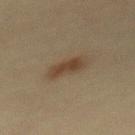Captured during whole-body skin photography for melanoma surveillance; the lesion was not biopsied. Longest diameter approximately 3.5 mm. This is a cross-polarized tile. A region of skin cropped from a whole-body photographic capture, roughly 15 mm wide. On the back. The subject is a female aged approximately 40. The total-body-photography lesion software estimated a mean CIELAB color near L≈34 a*≈13 b*≈25, a lesion–skin lightness drop of about 8, and a normalized border contrast of about 8. The software also gave a color-variation rating of about 1.5/10 and radial color variation of about 0.5. It also reported a classifier nevus-likeness of about 95/100 and a detector confidence of about 100 out of 100 that the crop contains a lesion.